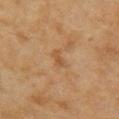Captured during whole-body skin photography for melanoma surveillance; the lesion was not biopsied. From the right upper arm. Measured at roughly 2.5 mm in maximum diameter. A female subject, in their 60s. The tile uses cross-polarized illumination. A lesion tile, about 15 mm wide, cut from a 3D total-body photograph.